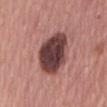The lesion was tiled from a total-body skin photograph and was not biopsied.
From the mid back.
A close-up tile cropped from a whole-body skin photograph, about 15 mm across.
This is a white-light tile.
A male patient, aged around 65.
Automated image analysis of the tile measured a footprint of about 19 mm² and an eccentricity of roughly 0.65. The analysis additionally found a border-irregularity index near 1.5/10, a within-lesion color-variation index near 5/10, and radial color variation of about 1.5.